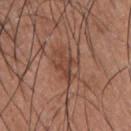Case summary:
• follow-up — total-body-photography surveillance lesion; no biopsy
• size — ≈4.5 mm
• image source — 15 mm crop, total-body photography
• subject — male, aged approximately 35
• lighting — white-light illumination
• site — the front of the torso